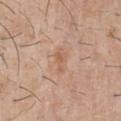Assessment:
Recorded during total-body skin imaging; not selected for excision or biopsy.
Image and clinical context:
The patient is a male roughly 30 years of age. This image is a 15 mm lesion crop taken from a total-body photograph. Located on the chest. The recorded lesion diameter is about 3 mm.